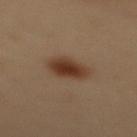location — the back | imaging modality — total-body-photography crop, ~15 mm field of view | subject — female, aged 48 to 52.